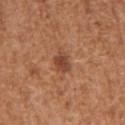workup: total-body-photography surveillance lesion; no biopsy
image-analysis metrics: a border-irregularity index near 2/10, internal color variation of about 2.5 on a 0–10 scale, and peripheral color asymmetry of about 1; an automated nevus-likeness rating near 50 out of 100 and lesion-presence confidence of about 100/100
body site: the arm
subject: female, aged approximately 40
image source: ~15 mm crop, total-body skin-cancer survey
tile lighting: white-light illumination